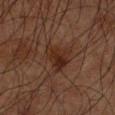This lesion was catalogued during total-body skin photography and was not selected for biopsy. The lesion is on the left upper arm. A male patient, in their mid-60s. Automated tile analysis of the lesion measured an average lesion color of about L≈22 a*≈16 b*≈22 (CIELAB), a lesion–skin lightness drop of about 6, and a normalized border contrast of about 7.5. It also reported a color-variation rating of about 4/10 and radial color variation of about 1.5. The analysis additionally found a classifier nevus-likeness of about 40/100 and a lesion-detection confidence of about 100/100. Captured under cross-polarized illumination. This image is a 15 mm lesion crop taken from a total-body photograph. Measured at roughly 4 mm in maximum diameter.